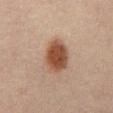follow-up: no biopsy performed (imaged during a skin exam)
lighting: cross-polarized
subject: male, aged around 30
size: ~4.5 mm (longest diameter)
acquisition: 15 mm crop, total-body photography
anatomic site: the abdomen
automated lesion analysis: a footprint of about 12 mm², a shape eccentricity near 0.7, and two-axis asymmetry of about 0.1; a mean CIELAB color near L≈40 a*≈18 b*≈26 and a lesion–skin lightness drop of about 12